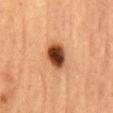Impression: Part of a total-body skin-imaging series; this lesion was reviewed on a skin check and was not flagged for biopsy. Background: The lesion is on the abdomen. A male subject in their mid-80s or older. Cropped from a whole-body photographic skin survey; the tile spans about 15 mm.